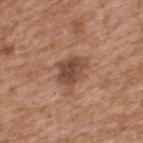Image and clinical context: An algorithmic analysis of the crop reported an average lesion color of about L≈47 a*≈21 b*≈28 (CIELAB) and roughly 11 lightness units darker than nearby skin. And it measured a border-irregularity index near 3/10, a color-variation rating of about 4/10, and a peripheral color-asymmetry measure near 1.5. A male subject, in their mid- to late 60s. Captured under white-light illumination. The recorded lesion diameter is about 4 mm. The lesion is on the upper back. A 15 mm close-up tile from a total-body photography series done for melanoma screening.